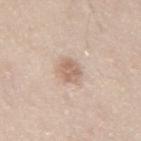Assessment:
Imaged during a routine full-body skin examination; the lesion was not biopsied and no histopathology is available.
Background:
A male subject roughly 45 years of age. Located on the mid back. A roughly 15 mm field-of-view crop from a total-body skin photograph.